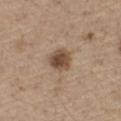Q: Is there a histopathology result?
A: total-body-photography surveillance lesion; no biopsy
Q: Patient demographics?
A: male, approximately 70 years of age
Q: Where on the body is the lesion?
A: the left thigh
Q: How was the tile lit?
A: white-light illumination
Q: How was this image acquired?
A: 15 mm crop, total-body photography
Q: How large is the lesion?
A: about 3 mm